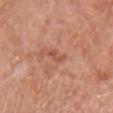patient = female, aged approximately 60; imaging modality = ~15 mm tile from a whole-body skin photo; anatomic site = the chest.